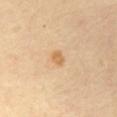<case>
  <biopsy_status>not biopsied; imaged during a skin examination</biopsy_status>
  <site>abdomen</site>
  <lighting>cross-polarized</lighting>
  <image>
    <source>total-body photography crop</source>
    <field_of_view_mm>15</field_of_view_mm>
  </image>
  <patient>
    <sex>female</sex>
    <age_approx>65</age_approx>
  </patient>
</case>